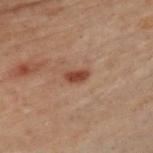Q: Was a biopsy performed?
A: no biopsy performed (imaged during a skin exam)
Q: How was this image acquired?
A: total-body-photography crop, ~15 mm field of view
Q: Lesion location?
A: the front of the torso
Q: Who is the patient?
A: male, approximately 50 years of age